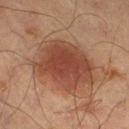Captured during whole-body skin photography for melanoma surveillance; the lesion was not biopsied. Imaged with cross-polarized lighting. The subject is a male approximately 65 years of age. The recorded lesion diameter is about 7.5 mm. The total-body-photography lesion software estimated about 10 CIELAB-L* units darker than the surrounding skin and a lesion-to-skin contrast of about 8.5 (normalized; higher = more distinct). The analysis additionally found a border-irregularity index near 2.5/10 and peripheral color asymmetry of about 1. It also reported a detector confidence of about 100 out of 100 that the crop contains a lesion. The lesion is located on the left thigh. This image is a 15 mm lesion crop taken from a total-body photograph.